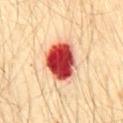Q: Is there a histopathology result?
A: total-body-photography surveillance lesion; no biopsy
Q: Lesion location?
A: the front of the torso
Q: What did automated image analysis measure?
A: a footprint of about 17 mm², an eccentricity of roughly 0.7, and a shape-asymmetry score of about 0.25 (0 = symmetric); a nevus-likeness score of about 0/100 and a detector confidence of about 100 out of 100 that the crop contains a lesion
Q: Who is the patient?
A: male, in their 30s
Q: How was this image acquired?
A: ~15 mm tile from a whole-body skin photo
Q: How large is the lesion?
A: about 5.5 mm